The total-body-photography lesion software estimated a lesion area of about 16 mm², a shape eccentricity near 0.6, and a symmetry-axis asymmetry near 0.3. The software also gave border irregularity of about 3.5 on a 0–10 scale, internal color variation of about 6.5 on a 0–10 scale, and a peripheral color-asymmetry measure near 2.5. It also reported a nevus-likeness score of about 45/100 and a detector confidence of about 100 out of 100 that the crop contains a lesion · a lesion tile, about 15 mm wide, cut from a 3D total-body photograph · a male subject, aged 63–67 · about 5 mm across · from the left lower leg.
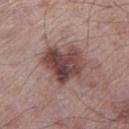pathology=an invasive melanoma, superficial spreading type; Breslow depth 0.3 mm, mitotic rate <1/mm² — a malignant lesion